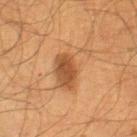Findings:
• workup: no biopsy performed (imaged during a skin exam)
• body site: the right thigh
• subject: male, about 60 years old
• illumination: cross-polarized
• image: ~15 mm tile from a whole-body skin photo
• lesion size: about 6 mm